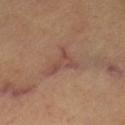Impression:
The lesion was tiled from a total-body skin photograph and was not biopsied.
Background:
A 15 mm close-up extracted from a 3D total-body photography capture. On the left thigh. Longest diameter approximately 4.5 mm. A subject in their 60s. Captured under cross-polarized illumination.